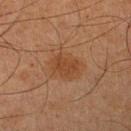Clinical impression:
No biopsy was performed on this lesion — it was imaged during a full skin examination and was not determined to be concerning.
Clinical summary:
Captured under cross-polarized illumination. This image is a 15 mm lesion crop taken from a total-body photograph. Located on the leg. The patient is a male roughly 65 years of age. The lesion-visualizer software estimated an average lesion color of about L≈36 a*≈18 b*≈29 (CIELAB), about 6 CIELAB-L* units darker than the surrounding skin, and a normalized border contrast of about 6. The analysis additionally found border irregularity of about 3 on a 0–10 scale and peripheral color asymmetry of about 0.5. It also reported an automated nevus-likeness rating near 15 out of 100 and lesion-presence confidence of about 100/100.